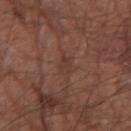Assessment: This lesion was catalogued during total-body skin photography and was not selected for biopsy. Background: The recorded lesion diameter is about 2.5 mm. Imaged with white-light lighting. The lesion is located on the right forearm. A roughly 15 mm field-of-view crop from a total-body skin photograph. A male patient roughly 65 years of age.